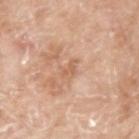Captured during whole-body skin photography for melanoma surveillance; the lesion was not biopsied. A region of skin cropped from a whole-body photographic capture, roughly 15 mm wide. Measured at roughly 2.5 mm in maximum diameter. Automated tile analysis of the lesion measured an area of roughly 3 mm², a shape eccentricity near 0.85, and two-axis asymmetry of about 0.35. It also reported a lesion color around L≈62 a*≈22 b*≈33 in CIELAB, about 8 CIELAB-L* units darker than the surrounding skin, and a lesion-to-skin contrast of about 5.5 (normalized; higher = more distinct). And it measured a nevus-likeness score of about 0/100 and lesion-presence confidence of about 100/100. A female patient roughly 75 years of age. This is a white-light tile. Located on the left upper arm.